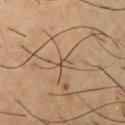The lesion was tiled from a total-body skin photograph and was not biopsied. A male subject, in their mid- to late 50s. On the chest. A region of skin cropped from a whole-body photographic capture, roughly 15 mm wide. This is a cross-polarized tile. The total-body-photography lesion software estimated a lesion area of about 1 mm². The software also gave a border-irregularity index near 4/10, a within-lesion color-variation index near 0/10, and peripheral color asymmetry of about 0. The recorded lesion diameter is about 1.5 mm.